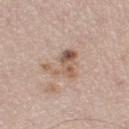Clinical impression: The lesion was photographed on a routine skin check and not biopsied; there is no pathology result. Clinical summary: A close-up tile cropped from a whole-body skin photograph, about 15 mm across. An algorithmic analysis of the crop reported a lesion area of about 8.5 mm², a shape eccentricity near 0.55, and a symmetry-axis asymmetry near 0.6. It also reported an average lesion color of about L≈57 a*≈17 b*≈28 (CIELAB), about 10 CIELAB-L* units darker than the surrounding skin, and a normalized border contrast of about 7.5. And it measured border irregularity of about 8.5 on a 0–10 scale and a peripheral color-asymmetry measure near 3. Imaged with white-light lighting. Measured at roughly 4 mm in maximum diameter. The lesion is on the right thigh. A male patient aged 58–62.